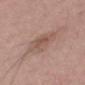Impression:
Captured during whole-body skin photography for melanoma surveillance; the lesion was not biopsied.
Image and clinical context:
A male patient about 40 years old. A 15 mm crop from a total-body photograph taken for skin-cancer surveillance. Imaged with white-light lighting. The lesion is located on the mid back.